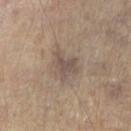Imaged during a routine full-body skin examination; the lesion was not biopsied and no histopathology is available. Cropped from a whole-body photographic skin survey; the tile spans about 15 mm. Imaged with white-light lighting. A male subject, approximately 60 years of age. On the right forearm. An algorithmic analysis of the crop reported an area of roughly 6.5 mm² and an eccentricity of roughly 0.65. It also reported a color-variation rating of about 1.5/10 and a peripheral color-asymmetry measure near 0.5. The software also gave a classifier nevus-likeness of about 0/100.